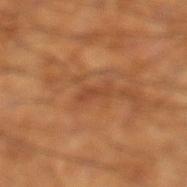notes: no biopsy performed (imaged during a skin exam) | illumination: cross-polarized | lesion size: about 3 mm | imaging modality: total-body-photography crop, ~15 mm field of view | automated metrics: a lesion area of about 2 mm², a shape eccentricity near 0.95, and a shape-asymmetry score of about 0.5 (0 = symmetric); a lesion color around L≈44 a*≈25 b*≈35 in CIELAB, roughly 7 lightness units darker than nearby skin, and a lesion-to-skin contrast of about 6 (normalized; higher = more distinct); an automated nevus-likeness rating near 0 out of 100 and a detector confidence of about 75 out of 100 that the crop contains a lesion | subject: male, in their 60s | site: the leg.